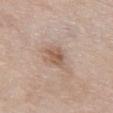notes: total-body-photography surveillance lesion; no biopsy | location: the chest | illumination: white-light | acquisition: ~15 mm tile from a whole-body skin photo | automated lesion analysis: an area of roughly 5 mm² and an eccentricity of roughly 0.3; an average lesion color of about L≈56 a*≈18 b*≈28 (CIELAB); a nevus-likeness score of about 15/100 and lesion-presence confidence of about 100/100 | subject: female, in their mid-60s | lesion size: ≈2.5 mm.